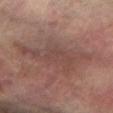Captured during whole-body skin photography for melanoma surveillance; the lesion was not biopsied. On the left arm. A 15 mm close-up tile from a total-body photography series done for melanoma screening. This is a cross-polarized tile. A female subject, aged 78 to 82.